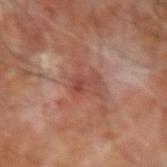Clinical impression: Recorded during total-body skin imaging; not selected for excision or biopsy. Context: The tile uses cross-polarized illumination. The lesion is located on the right forearm. A male patient, aged 63 to 67. Cropped from a total-body skin-imaging series; the visible field is about 15 mm. Longest diameter approximately 3 mm.